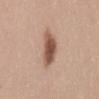<lesion>
  <biopsy_status>not biopsied; imaged during a skin examination</biopsy_status>
  <lighting>white-light</lighting>
  <image>
    <source>total-body photography crop</source>
    <field_of_view_mm>15</field_of_view_mm>
  </image>
  <patient>
    <sex>female</sex>
    <age_approx>35</age_approx>
  </patient>
  <automated_metrics>
    <area_mm2_approx>9.5</area_mm2_approx>
    <eccentricity>0.8</eccentricity>
    <shape_asymmetry>0.25</shape_asymmetry>
  </automated_metrics>
  <lesion_size>
    <long_diameter_mm_approx>4.5</long_diameter_mm_approx>
  </lesion_size>
  <site>lower back</site>
</lesion>